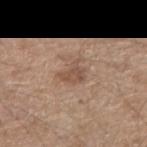biopsy_status: not biopsied; imaged during a skin examination
automated_metrics:
  area_mm2_approx: 5.0
  shape_asymmetry: 0.45
  cielab_L: 51
  cielab_a: 18
  cielab_b: 28
  vs_skin_darker_L: 8.0
  vs_skin_contrast_norm: 6.0
  border_irregularity_0_10: 4.5
  color_variation_0_10: 2.0
  peripheral_color_asymmetry: 0.5
  nevus_likeness_0_100: 10
  lesion_detection_confidence_0_100: 100
lighting: white-light
image:
  source: total-body photography crop
  field_of_view_mm: 15
patient:
  sex: male
  age_approx: 65
lesion_size:
  long_diameter_mm_approx: 3.0
site: right upper arm